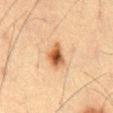follow-up: catalogued during a skin exam; not biopsied | diameter: about 3 mm | body site: the front of the torso | image source: ~15 mm crop, total-body skin-cancer survey | patient: male, aged approximately 60 | illumination: cross-polarized.